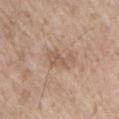patient:
  sex: male
  age_approx: 70
site: right upper arm
image:
  source: total-body photography crop
  field_of_view_mm: 15